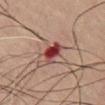The lesion was tiled from a total-body skin photograph and was not biopsied. The lesion is located on the front of the torso. A region of skin cropped from a whole-body photographic capture, roughly 15 mm wide. The total-body-photography lesion software estimated a lesion–skin lightness drop of about 14 and a normalized lesion–skin contrast near 12. The patient is a male in their 60s. Approximately 3 mm at its widest. This is a cross-polarized tile.